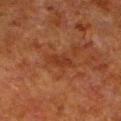| key | value |
|---|---|
| acquisition | 15 mm crop, total-body photography |
| anatomic site | the left lower leg |
| subject | male, in their 80s |